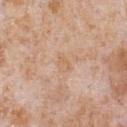Cropped from a total-body skin-imaging series; the visible field is about 15 mm.
The tile uses white-light illumination.
Longest diameter approximately 2.5 mm.
On the front of the torso.
A male subject aged around 65.